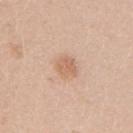Q: Was a biopsy performed?
A: total-body-photography surveillance lesion; no biopsy
Q: What is the lesion's diameter?
A: ≈2.5 mm
Q: What is the imaging modality?
A: ~15 mm tile from a whole-body skin photo
Q: How was the tile lit?
A: white-light
Q: What are the patient's age and sex?
A: female, aged 18 to 22
Q: Where on the body is the lesion?
A: the arm
Q: Automated lesion metrics?
A: a footprint of about 5.5 mm², an eccentricity of roughly 0.55, and a shape-asymmetry score of about 0.2 (0 = symmetric); a mean CIELAB color near L≈64 a*≈20 b*≈32, about 9 CIELAB-L* units darker than the surrounding skin, and a normalized border contrast of about 6; a border-irregularity index near 2/10, internal color variation of about 2 on a 0–10 scale, and peripheral color asymmetry of about 0.5